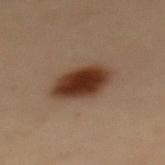Q: Was this lesion biopsied?
A: total-body-photography surveillance lesion; no biopsy
Q: What are the patient's age and sex?
A: female, in their 50s
Q: How large is the lesion?
A: about 4.5 mm
Q: What did automated image analysis measure?
A: a lesion area of about 15 mm², an outline eccentricity of about 0.65 (0 = round, 1 = elongated), and a symmetry-axis asymmetry near 0.15; a mean CIELAB color near L≈30 a*≈17 b*≈25, roughly 14 lightness units darker than nearby skin, and a normalized lesion–skin contrast near 13
Q: What is the anatomic site?
A: the mid back
Q: What kind of image is this?
A: ~15 mm crop, total-body skin-cancer survey
Q: Illumination type?
A: cross-polarized illumination